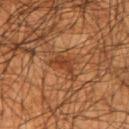biopsy status — total-body-photography surveillance lesion; no biopsy